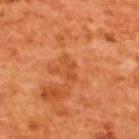Impression: Captured during whole-body skin photography for melanoma surveillance; the lesion was not biopsied. Clinical summary: Captured under cross-polarized illumination. From the upper back. Approximately 3 mm at its widest. A 15 mm close-up extracted from a 3D total-body photography capture. A male subject, approximately 65 years of age.